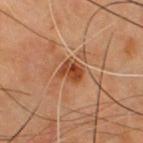biopsy status — catalogued during a skin exam; not biopsied